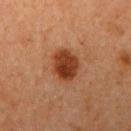Q: Was this lesion biopsied?
A: catalogued during a skin exam; not biopsied
Q: Lesion location?
A: the right upper arm
Q: What did automated image analysis measure?
A: an average lesion color of about L≈32 a*≈22 b*≈30 (CIELAB) and a normalized lesion–skin contrast near 10.5
Q: Who is the patient?
A: female, about 60 years old
Q: How large is the lesion?
A: ≈4 mm
Q: What kind of image is this?
A: ~15 mm crop, total-body skin-cancer survey